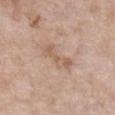Recorded during total-body skin imaging; not selected for excision or biopsy. Captured under white-light illumination. Approximately 4.5 mm at its widest. An algorithmic analysis of the crop reported a lesion–skin lightness drop of about 8 and a normalized lesion–skin contrast near 5.5. And it measured a nevus-likeness score of about 0/100 and lesion-presence confidence of about 100/100. From the chest. A 15 mm close-up extracted from a 3D total-body photography capture. A female patient about 75 years old.